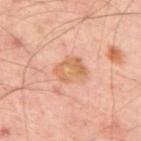A 15 mm crop from a total-body photograph taken for skin-cancer surveillance.
Captured under cross-polarized illumination.
From the mid back.
The total-body-photography lesion software estimated an area of roughly 8.5 mm², an eccentricity of roughly 0.65, and a symmetry-axis asymmetry near 0.25. The analysis additionally found a border-irregularity index near 2.5/10 and a peripheral color-asymmetry measure near 1.5.
A patient aged approximately 55.